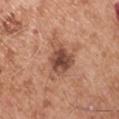No biopsy was performed on this lesion — it was imaged during a full skin examination and was not determined to be concerning.
This is a white-light tile.
The lesion is on the left upper arm.
A lesion tile, about 15 mm wide, cut from a 3D total-body photograph.
The subject is a male aged around 55.
An algorithmic analysis of the crop reported a shape eccentricity near 0.7 and a symmetry-axis asymmetry near 0.45. The software also gave a lesion–skin lightness drop of about 13 and a normalized lesion–skin contrast near 9. The software also gave a border-irregularity rating of about 5/10 and a color-variation rating of about 5.5/10. The software also gave a nevus-likeness score of about 70/100 and a lesion-detection confidence of about 100/100.
Approximately 5.5 mm at its widest.